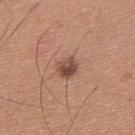{"biopsy_status": "not biopsied; imaged during a skin examination", "image": {"source": "total-body photography crop", "field_of_view_mm": 15}, "lighting": "white-light", "patient": {"sex": "male", "age_approx": 35}, "site": "upper back", "lesion_size": {"long_diameter_mm_approx": 2.5}}